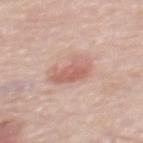Case summary:
– notes — no biopsy performed (imaged during a skin exam)
– acquisition — 15 mm crop, total-body photography
– lesion diameter — ≈4 mm
– location — the upper back
– patient — female, in their mid-60s
– TBP lesion metrics — a footprint of about 10 mm², a shape eccentricity near 0.7, and a symmetry-axis asymmetry near 0.25; an average lesion color of about L≈62 a*≈23 b*≈26 (CIELAB), about 10 CIELAB-L* units darker than the surrounding skin, and a lesion-to-skin contrast of about 6 (normalized; higher = more distinct)
– lighting — white-light